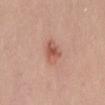Q: Was a biopsy performed?
A: imaged on a skin check; not biopsied
Q: How large is the lesion?
A: ≈3 mm
Q: What lighting was used for the tile?
A: white-light
Q: What kind of image is this?
A: ~15 mm crop, total-body skin-cancer survey
Q: What is the anatomic site?
A: the abdomen
Q: Patient demographics?
A: female, aged approximately 35